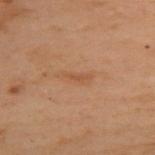{"biopsy_status": "not biopsied; imaged during a skin examination", "image": {"source": "total-body photography crop", "field_of_view_mm": 15}, "patient": {"sex": "female", "age_approx": 55}, "lighting": "cross-polarized", "lesion_size": {"long_diameter_mm_approx": 3.5}, "site": "upper back", "automated_metrics": {"area_mm2_approx": 3.5, "eccentricity": 0.95, "shape_asymmetry": 0.4, "nevus_likeness_0_100": 0, "lesion_detection_confidence_0_100": 100}}